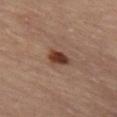Q: Was this lesion biopsied?
A: no biopsy performed (imaged during a skin exam)
Q: Patient demographics?
A: female, in their mid-60s
Q: How was this image acquired?
A: ~15 mm crop, total-body skin-cancer survey
Q: What lighting was used for the tile?
A: cross-polarized illumination
Q: What is the anatomic site?
A: the left thigh
Q: How large is the lesion?
A: ≈3 mm